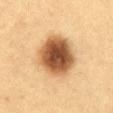Assessment: Imaged during a routine full-body skin examination; the lesion was not biopsied and no histopathology is available. Acquisition and patient details: A lesion tile, about 15 mm wide, cut from a 3D total-body photograph. From the abdomen. A female subject aged around 30.